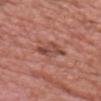Captured during whole-body skin photography for melanoma surveillance; the lesion was not biopsied. A male patient, aged 58–62. On the head or neck. Approximately 4 mm at its widest. A close-up tile cropped from a whole-body skin photograph, about 15 mm across.